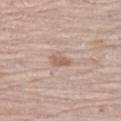illumination: white-light
location: the left thigh
size: about 2.5 mm
automated metrics: a lesion color around L≈60 a*≈17 b*≈27 in CIELAB and a normalized lesion–skin contrast near 6.5; border irregularity of about 3 on a 0–10 scale, internal color variation of about 1.5 on a 0–10 scale, and radial color variation of about 0.5; a lesion-detection confidence of about 100/100
patient: female, in their mid-60s
image: 15 mm crop, total-body photography